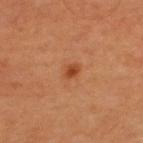Findings:
• biopsy status · no biopsy performed (imaged during a skin exam)
• image · ~15 mm crop, total-body skin-cancer survey
• illumination · cross-polarized
• anatomic site · the upper back
• lesion size · ~2 mm (longest diameter)
• image-analysis metrics · an area of roughly 2.5 mm², a shape eccentricity near 0.65, and a shape-asymmetry score of about 0.15 (0 = symmetric); a classifier nevus-likeness of about 90/100 and a lesion-detection confidence of about 100/100
• subject · male, aged approximately 65